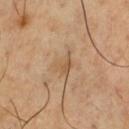<tbp_lesion>
  <biopsy_status>not biopsied; imaged during a skin examination</biopsy_status>
</tbp_lesion>